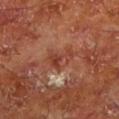Findings:
* automated metrics: a footprint of about 6 mm², a shape eccentricity near 0.7, and a shape-asymmetry score of about 0.45 (0 = symmetric); a border-irregularity rating of about 5/10, a within-lesion color-variation index near 6.5/10, and peripheral color asymmetry of about 2
* image source: ~15 mm crop, total-body skin-cancer survey
* location: the left lower leg
* tile lighting: cross-polarized illumination
* subject: male, in their mid- to late 60s
* size: ≈3.5 mm